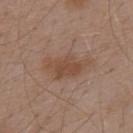Case summary:
• body site · the back
• tile lighting · white-light
• imaging modality · 15 mm crop, total-body photography
• lesion diameter · about 6 mm
• patient · male, in their mid-50s
• automated lesion analysis · an area of roughly 13 mm², a shape eccentricity near 0.85, and a shape-asymmetry score of about 0.35 (0 = symmetric)